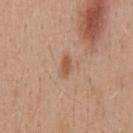{"biopsy_status": "not biopsied; imaged during a skin examination", "lesion_size": {"long_diameter_mm_approx": 2.5}, "automated_metrics": {"area_mm2_approx": 3.0, "eccentricity": 0.85, "shape_asymmetry": 0.35, "cielab_L": 54, "cielab_a": 21, "cielab_b": 32, "vs_skin_darker_L": 9.0, "nevus_likeness_0_100": 75, "lesion_detection_confidence_0_100": 100}, "lighting": "white-light", "site": "mid back", "patient": {"sex": "male", "age_approx": 40}, "image": {"source": "total-body photography crop", "field_of_view_mm": 15}}